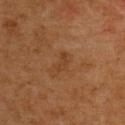Notes:
* lesion diameter: about 2.5 mm
* image: total-body-photography crop, ~15 mm field of view
* site: the upper back
* subject: male, aged 63 to 67
* TBP lesion metrics: an area of roughly 2 mm², an eccentricity of roughly 0.9, and a shape-asymmetry score of about 0.45 (0 = symmetric); border irregularity of about 5 on a 0–10 scale